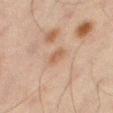Q: Is there a histopathology result?
A: no biopsy performed (imaged during a skin exam)
Q: Lesion size?
A: about 2.5 mm
Q: What is the imaging modality?
A: ~15 mm tile from a whole-body skin photo
Q: Where on the body is the lesion?
A: the right thigh
Q: Who is the patient?
A: male, aged around 45